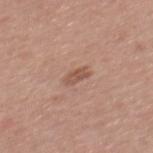No biopsy was performed on this lesion — it was imaged during a full skin examination and was not determined to be concerning. Captured under white-light illumination. The lesion is located on the mid back. A female subject aged around 35. The total-body-photography lesion software estimated a classifier nevus-likeness of about 5/100. Longest diameter approximately 2.5 mm. A region of skin cropped from a whole-body photographic capture, roughly 15 mm wide.